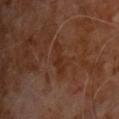* location — the chest
* size — ≈3.5 mm
* image source — total-body-photography crop, ~15 mm field of view
* automated lesion analysis — a footprint of about 4.5 mm², an outline eccentricity of about 0.9 (0 = round, 1 = elongated), and a symmetry-axis asymmetry near 0.4; a lesion color around L≈28 a*≈20 b*≈27 in CIELAB and a lesion–skin lightness drop of about 5
* illumination — cross-polarized illumination
* patient — male, roughly 60 years of age